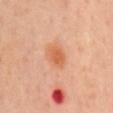Captured during whole-body skin photography for melanoma surveillance; the lesion was not biopsied.
A male patient in their mid-50s.
Automated image analysis of the tile measured a lesion area of about 6 mm² and a shape-asymmetry score of about 0.2 (0 = symmetric). The software also gave an average lesion color of about L≈61 a*≈27 b*≈39 (CIELAB), a lesion–skin lightness drop of about 9, and a lesion-to-skin contrast of about 7.5 (normalized; higher = more distinct). And it measured a classifier nevus-likeness of about 90/100 and a lesion-detection confidence of about 100/100.
A 15 mm crop from a total-body photograph taken for skin-cancer surveillance.
The lesion is located on the mid back.